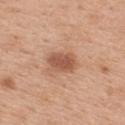Q: Where on the body is the lesion?
A: the upper back
Q: Who is the patient?
A: female, aged 38–42
Q: What is the imaging modality?
A: ~15 mm tile from a whole-body skin photo